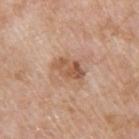<tbp_lesion>
  <biopsy_status>not biopsied; imaged during a skin examination</biopsy_status>
  <lighting>white-light</lighting>
  <patient>
    <sex>male</sex>
    <age_approx>60</age_approx>
  </patient>
  <site>upper back</site>
  <lesion_size>
    <long_diameter_mm_approx>3.5</long_diameter_mm_approx>
  </lesion_size>
  <image>
    <source>total-body photography crop</source>
    <field_of_view_mm>15</field_of_view_mm>
  </image>
</tbp_lesion>